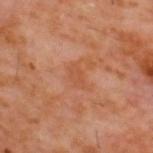Clinical impression:
No biopsy was performed on this lesion — it was imaged during a full skin examination and was not determined to be concerning.
Acquisition and patient details:
Measured at roughly 2.5 mm in maximum diameter. The lesion is located on the back. An algorithmic analysis of the crop reported a border-irregularity index near 2/10 and internal color variation of about 1 on a 0–10 scale. A male subject in their 60s. Captured under cross-polarized illumination. A close-up tile cropped from a whole-body skin photograph, about 15 mm across.